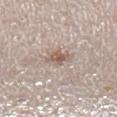<lesion>
<biopsy_status>not biopsied; imaged during a skin examination</biopsy_status>
</lesion>